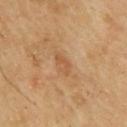Recorded during total-body skin imaging; not selected for excision or biopsy.
Approximately 3 mm at its widest.
A male subject, aged around 70.
Imaged with cross-polarized lighting.
This image is a 15 mm lesion crop taken from a total-body photograph.
The lesion is on the upper back.
An algorithmic analysis of the crop reported a lesion area of about 3 mm² and an eccentricity of roughly 0.9. And it measured roughly 7 lightness units darker than nearby skin and a lesion-to-skin contrast of about 5 (normalized; higher = more distinct).